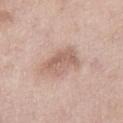Captured during whole-body skin photography for melanoma surveillance; the lesion was not biopsied. The subject is a female aged approximately 55. On the left thigh. Cropped from a whole-body photographic skin survey; the tile spans about 15 mm.